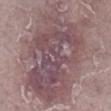{
  "biopsy_status": "not biopsied; imaged during a skin examination",
  "site": "right lower leg",
  "automated_metrics": {
    "cielab_L": 49,
    "cielab_a": 18,
    "cielab_b": 14,
    "vs_skin_contrast_norm": 7.5,
    "nevus_likeness_0_100": 0,
    "lesion_detection_confidence_0_100": 80
  },
  "image": {
    "source": "total-body photography crop",
    "field_of_view_mm": 15
  },
  "patient": {
    "sex": "female",
    "age_approx": 60
  },
  "lesion_size": {
    "long_diameter_mm_approx": 12.0
  },
  "lighting": "white-light"
}